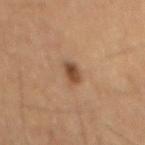Imaged during a routine full-body skin examination; the lesion was not biopsied and no histopathology is available. Measured at roughly 3 mm in maximum diameter. The lesion-visualizer software estimated an automated nevus-likeness rating near 95 out of 100 and a detector confidence of about 100 out of 100 that the crop contains a lesion. A 15 mm close-up tile from a total-body photography series done for melanoma screening. The patient is a male aged around 60.